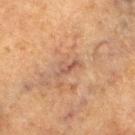Assessment: No biopsy was performed on this lesion — it was imaged during a full skin examination and was not determined to be concerning. Context: A male subject, in their mid-70s. A 15 mm crop from a total-body photograph taken for skin-cancer surveillance. The lesion is located on the right thigh.